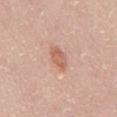<record>
  <biopsy_status>not biopsied; imaged during a skin examination</biopsy_status>
  <image>
    <source>total-body photography crop</source>
    <field_of_view_mm>15</field_of_view_mm>
  </image>
  <lighting>white-light</lighting>
  <patient>
    <sex>male</sex>
    <age_approx>50</age_approx>
  </patient>
  <lesion_size>
    <long_diameter_mm_approx>3.5</long_diameter_mm_approx>
  </lesion_size>
  <site>abdomen</site>
</record>